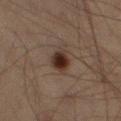{"biopsy_status": "not biopsied; imaged during a skin examination", "lighting": "cross-polarized", "patient": {"sex": "male", "age_approx": 55}, "site": "right thigh", "image": {"source": "total-body photography crop", "field_of_view_mm": 15}, "automated_metrics": {"eccentricity": 0.6, "shape_asymmetry": 0.15, "cielab_L": 25, "cielab_a": 14, "cielab_b": 20, "vs_skin_darker_L": 12.0, "vs_skin_contrast_norm": 12.5}}